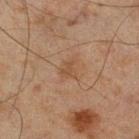Imaged during a routine full-body skin examination; the lesion was not biopsied and no histopathology is available.
An algorithmic analysis of the crop reported an outline eccentricity of about 0.65 (0 = round, 1 = elongated) and a shape-asymmetry score of about 0.3 (0 = symmetric). The software also gave about 5 CIELAB-L* units darker than the surrounding skin and a normalized border contrast of about 5.
A roughly 15 mm field-of-view crop from a total-body skin photograph.
From the right lower leg.
The recorded lesion diameter is about 3 mm.
A male patient aged 43–47.